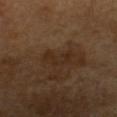Captured during whole-body skin photography for melanoma surveillance; the lesion was not biopsied.
The total-body-photography lesion software estimated a normalized border contrast of about 6. And it measured border irregularity of about 7.5 on a 0–10 scale, a color-variation rating of about 0/10, and radial color variation of about 0.
The lesion is located on the head or neck.
Captured under cross-polarized illumination.
Cropped from a whole-body photographic skin survey; the tile spans about 15 mm.
Longest diameter approximately 3.5 mm.
The subject is a female aged 63 to 67.